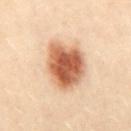workup=imaged on a skin check; not biopsied
body site=the abdomen
subject=female, aged 28–32
illumination=cross-polarized
size=~6 mm (longest diameter)
acquisition=~15 mm crop, total-body skin-cancer survey